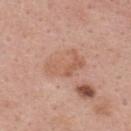{
  "biopsy_status": "not biopsied; imaged during a skin examination",
  "site": "upper back",
  "lesion_size": {
    "long_diameter_mm_approx": 4.5
  },
  "image": {
    "source": "total-body photography crop",
    "field_of_view_mm": 15
  },
  "patient": {
    "sex": "female",
    "age_approx": 35
  },
  "lighting": "white-light"
}